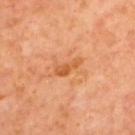<record>
<biopsy_status>not biopsied; imaged during a skin examination</biopsy_status>
<lighting>cross-polarized</lighting>
<image>
  <source>total-body photography crop</source>
  <field_of_view_mm>15</field_of_view_mm>
</image>
<patient>
  <sex>male</sex>
  <age_approx>70</age_approx>
</patient>
<site>back</site>
<lesion_size>
  <long_diameter_mm_approx>3.0</long_diameter_mm_approx>
</lesion_size>
</record>